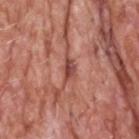Case summary:
• workup: catalogued during a skin exam; not biopsied
• subject: male, approximately 60 years of age
• acquisition: ~15 mm tile from a whole-body skin photo
• site: the head or neck